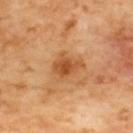* notes — catalogued during a skin exam; not biopsied